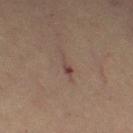Assessment: The lesion was tiled from a total-body skin photograph and was not biopsied. Context: Captured under cross-polarized illumination. The subject is a female roughly 70 years of age. Located on the left thigh. The lesion-visualizer software estimated a mean CIELAB color near L≈37 a*≈16 b*≈18 and a normalized lesion–skin contrast near 6.5. Measured at roughly 3 mm in maximum diameter. A 15 mm crop from a total-body photograph taken for skin-cancer surveillance.